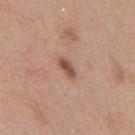Assessment: No biopsy was performed on this lesion — it was imaged during a full skin examination and was not determined to be concerning. Background: A female patient, aged around 40. A 15 mm close-up tile from a total-body photography series done for melanoma screening. On the back.